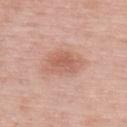Case summary:
* body site · the upper back
* patient · female, in their 50s
* image · 15 mm crop, total-body photography